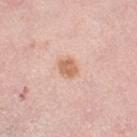Clinical impression:
The lesion was tiled from a total-body skin photograph and was not biopsied.
Background:
A female patient, in their mid-60s. The lesion is located on the left thigh. An algorithmic analysis of the crop reported a symmetry-axis asymmetry near 0.2. It also reported a border-irregularity rating of about 2/10, a within-lesion color-variation index near 1.5/10, and peripheral color asymmetry of about 0.5. A region of skin cropped from a whole-body photographic capture, roughly 15 mm wide. This is a white-light tile.